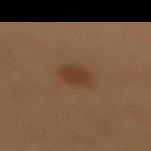Assessment: Captured during whole-body skin photography for melanoma surveillance; the lesion was not biopsied. Acquisition and patient details: This is a cross-polarized tile. A female patient aged 48 to 52. About 3 mm across. This image is a 15 mm lesion crop taken from a total-body photograph. From the upper back. Automated tile analysis of the lesion measured a lesion area of about 5.5 mm², an eccentricity of roughly 0.65, and a shape-asymmetry score of about 0.15 (0 = symmetric). And it measured a border-irregularity index near 1.5/10, a within-lesion color-variation index near 1.5/10, and a peripheral color-asymmetry measure near 0.5.